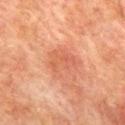Q: Lesion location?
A: the back
Q: What are the patient's age and sex?
A: male, about 75 years old
Q: What kind of image is this?
A: ~15 mm crop, total-body skin-cancer survey